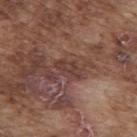notes = no biopsy performed (imaged during a skin exam); lesion diameter = ~3.5 mm (longest diameter); automated metrics = a footprint of about 5 mm² and two-axis asymmetry of about 0.25; lighting = white-light; patient = male, about 75 years old; image = 15 mm crop, total-body photography; body site = the upper back.